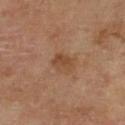Assessment: The lesion was tiled from a total-body skin photograph and was not biopsied.